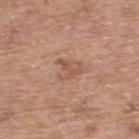Part of a total-body skin-imaging series; this lesion was reviewed on a skin check and was not flagged for biopsy. Located on the upper back. A 15 mm close-up extracted from a 3D total-body photography capture. The subject is a male aged approximately 55.